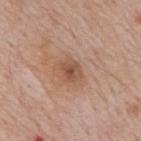This lesion was catalogued during total-body skin photography and was not selected for biopsy.
A male patient, about 60 years old.
From the back.
The tile uses white-light illumination.
Automated image analysis of the tile measured a classifier nevus-likeness of about 5/100.
About 3 mm across.
A close-up tile cropped from a whole-body skin photograph, about 15 mm across.